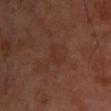follow-up: total-body-photography surveillance lesion; no biopsy | image-analysis metrics: an area of roughly 3 mm², an eccentricity of roughly 0.9, and two-axis asymmetry of about 0.5; a lesion color around L≈30 a*≈21 b*≈25 in CIELAB, roughly 4 lightness units darker than nearby skin, and a normalized lesion–skin contrast near 4.5; a border-irregularity index near 5.5/10 and radial color variation of about 0; a classifier nevus-likeness of about 0/100 | body site: the chest | subject: male, roughly 50 years of age | illumination: cross-polarized illumination | imaging modality: total-body-photography crop, ~15 mm field of view | size: ≈3 mm.